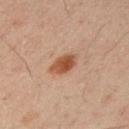| key | value |
|---|---|
| subject | male, in their 50s |
| tile lighting | cross-polarized illumination |
| site | the arm |
| automated metrics | a shape eccentricity near 0.75 and a symmetry-axis asymmetry near 0.1; an average lesion color of about L≈46 a*≈22 b*≈31 (CIELAB) and a lesion-to-skin contrast of about 9.5 (normalized; higher = more distinct); a within-lesion color-variation index near 4/10 and peripheral color asymmetry of about 1; a classifier nevus-likeness of about 100/100 and a lesion-detection confidence of about 100/100 |
| acquisition | ~15 mm crop, total-body skin-cancer survey |